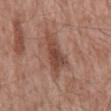Part of a total-body skin-imaging series; this lesion was reviewed on a skin check and was not flagged for biopsy. A male patient roughly 60 years of age. A 15 mm crop from a total-body photograph taken for skin-cancer surveillance. The lesion-visualizer software estimated a mean CIELAB color near L≈46 a*≈22 b*≈26, roughly 10 lightness units darker than nearby skin, and a lesion-to-skin contrast of about 8 (normalized; higher = more distinct). And it measured a border-irregularity rating of about 5/10, internal color variation of about 4 on a 0–10 scale, and a peripheral color-asymmetry measure near 1. It also reported a classifier nevus-likeness of about 5/100. The tile uses white-light illumination. The lesion is located on the mid back.